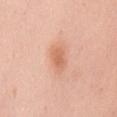<tbp_lesion>
  <image>
    <source>total-body photography crop</source>
    <field_of_view_mm>15</field_of_view_mm>
  </image>
  <automated_metrics>
    <eccentricity>0.8</eccentricity>
    <nevus_likeness_0_100>95</nevus_likeness_0_100>
    <lesion_detection_confidence_0_100>100</lesion_detection_confidence_0_100>
  </automated_metrics>
  <lighting>white-light</lighting>
  <site>mid back</site>
  <patient>
    <sex>female</sex>
    <age_approx>50</age_approx>
  </patient>
</tbp_lesion>